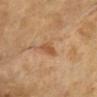Findings:
– workup · total-body-photography surveillance lesion; no biopsy
– body site · the head or neck
– image-analysis metrics · an area of roughly 3 mm², an outline eccentricity of about 0.9 (0 = round, 1 = elongated), and two-axis asymmetry of about 0.25
– imaging modality · 15 mm crop, total-body photography
– patient · female, aged 58 to 62
– lighting · cross-polarized